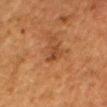Findings:
• follow-up — no biopsy performed (imaged during a skin exam)
• patient — female, approximately 50 years of age
• size — ≈2.5 mm
• image-analysis metrics — a mean CIELAB color near L≈36 a*≈22 b*≈31, roughly 7 lightness units darker than nearby skin, and a normalized lesion–skin contrast near 6.5; a classifier nevus-likeness of about 0/100 and a detector confidence of about 100 out of 100 that the crop contains a lesion
• imaging modality — ~15 mm crop, total-body skin-cancer survey
• location — the mid back